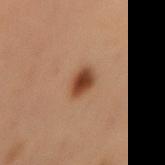- workup: catalogued during a skin exam; not biopsied
- anatomic site: the left thigh
- tile lighting: cross-polarized illumination
- patient: male, aged approximately 50
- imaging modality: ~15 mm tile from a whole-body skin photo
- automated metrics: an outline eccentricity of about 0.65 (0 = round, 1 = elongated) and a symmetry-axis asymmetry near 0.25; a nevus-likeness score of about 100/100
- diameter: about 3 mm